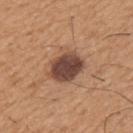– follow-up · no biopsy performed (imaged during a skin exam)
– site · the arm
– lesion diameter · about 4.5 mm
– imaging modality · total-body-photography crop, ~15 mm field of view
– patient · male, about 65 years old
– tile lighting · white-light